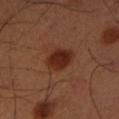Clinical impression: No biopsy was performed on this lesion — it was imaged during a full skin examination and was not determined to be concerning. Background: A 15 mm close-up tile from a total-body photography series done for melanoma screening. Approximately 3.5 mm at its widest. Located on the left lower leg. An algorithmic analysis of the crop reported a mean CIELAB color near L≈27 a*≈24 b*≈28 and roughly 10 lightness units darker than nearby skin. The patient is a male in their 50s.